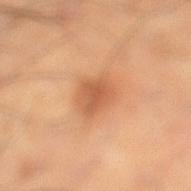follow-up — catalogued during a skin exam; not biopsied | image source — 15 mm crop, total-body photography | image-analysis metrics — a footprint of about 7.5 mm² and an outline eccentricity of about 0.35 (0 = round, 1 = elongated); an average lesion color of about L≈57 a*≈25 b*≈38 (CIELAB), a lesion–skin lightness drop of about 10, and a normalized lesion–skin contrast near 6.5; border irregularity of about 2.5 on a 0–10 scale and a within-lesion color-variation index near 3/10; a nevus-likeness score of about 55/100 and a detector confidence of about 100 out of 100 that the crop contains a lesion | tile lighting — cross-polarized illumination | site — the left lower leg | diameter — ~3.5 mm (longest diameter) | subject — male, aged 38–42.